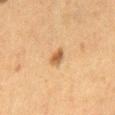workup: no biopsy performed (imaged during a skin exam)
subject: female, aged around 50
image source: 15 mm crop, total-body photography
illumination: cross-polarized illumination
size: ~2.5 mm (longest diameter)
site: the front of the torso